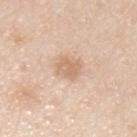workup — no biopsy performed (imaged during a skin exam); diameter — about 2.5 mm; patient — male, about 35 years old; lighting — white-light illumination; imaging modality — 15 mm crop, total-body photography; body site — the arm.